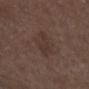* notes — total-body-photography surveillance lesion; no biopsy
* body site — the left lower leg
* automated metrics — an outline eccentricity of about 0.85 (0 = round, 1 = elongated) and two-axis asymmetry of about 0.45; roughly 5 lightness units darker than nearby skin and a lesion-to-skin contrast of about 5 (normalized; higher = more distinct); border irregularity of about 5 on a 0–10 scale and radial color variation of about 0.5
* diameter — about 3.5 mm
* subject — male, roughly 75 years of age
* lighting — white-light illumination
* image — ~15 mm crop, total-body skin-cancer survey